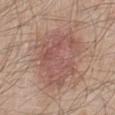Notes:
– biopsy status: imaged on a skin check; not biopsied
– tile lighting: white-light illumination
– patient: male, aged 58–62
– body site: the leg
– image source: 15 mm crop, total-body photography
– lesion size: about 7 mm